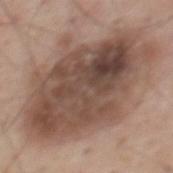Automated tile analysis of the lesion measured an area of roughly 75 mm², an outline eccentricity of about 0.8 (0 = round, 1 = elongated), and a shape-asymmetry score of about 0.2 (0 = symmetric). A region of skin cropped from a whole-body photographic capture, roughly 15 mm wide. From the mid back. The recorded lesion diameter is about 12.5 mm. A male subject aged 58–62. Captured under white-light illumination.